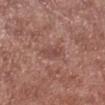Q: Was a biopsy performed?
A: imaged on a skin check; not biopsied
Q: Illumination type?
A: white-light illumination
Q: Who is the patient?
A: male, roughly 55 years of age
Q: Lesion location?
A: the left lower leg
Q: What kind of image is this?
A: 15 mm crop, total-body photography
Q: What is the lesion's diameter?
A: ≈3 mm